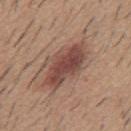Case summary:
* follow-up: catalogued during a skin exam; not biopsied
* lesion size: ≈7 mm
* automated lesion analysis: an area of roughly 18 mm², an outline eccentricity of about 0.85 (0 = round, 1 = elongated), and a shape-asymmetry score of about 0.25 (0 = symmetric); a lesion color around L≈46 a*≈21 b*≈25 in CIELAB, about 12 CIELAB-L* units darker than the surrounding skin, and a normalized lesion–skin contrast near 9
* site: the mid back
* imaging modality: total-body-photography crop, ~15 mm field of view
* lighting: white-light illumination
* subject: male, roughly 60 years of age